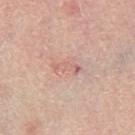Image and clinical context: Located on the right thigh. A female subject about 65 years old. A 15 mm crop from a total-body photograph taken for skin-cancer surveillance. The recorded lesion diameter is about 3.5 mm. This is a white-light tile.